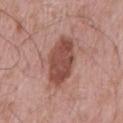- subject: male, aged 58 to 62
- size: ~6 mm (longest diameter)
- location: the back
- tile lighting: white-light
- image: ~15 mm tile from a whole-body skin photo